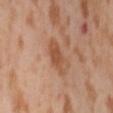From the lower back.
The lesion's longest dimension is about 4 mm.
A lesion tile, about 15 mm wide, cut from a 3D total-body photograph.
A female subject in their mid-50s.